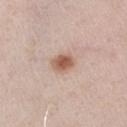Captured during whole-body skin photography for melanoma surveillance; the lesion was not biopsied. Longest diameter approximately 3 mm. Automated image analysis of the tile measured an average lesion color of about L≈58 a*≈19 b*≈29 (CIELAB) and a normalized lesion–skin contrast near 9. It also reported border irregularity of about 1.5 on a 0–10 scale. From the right upper arm. The patient is a male aged 68 to 72. This is a white-light tile. This image is a 15 mm lesion crop taken from a total-body photograph.